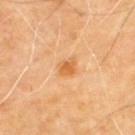workup: no biopsy performed (imaged during a skin exam).